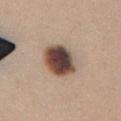Captured during whole-body skin photography for melanoma surveillance; the lesion was not biopsied.